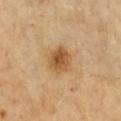Clinical impression:
This lesion was catalogued during total-body skin photography and was not selected for biopsy.
Acquisition and patient details:
A lesion tile, about 15 mm wide, cut from a 3D total-body photograph. This is a cross-polarized tile. The lesion is on the chest. A female subject, about 70 years old. The total-body-photography lesion software estimated an average lesion color of about L≈55 a*≈20 b*≈41 (CIELAB), a lesion–skin lightness drop of about 12, and a normalized lesion–skin contrast near 8.5.